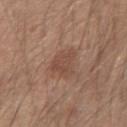Clinical impression: Part of a total-body skin-imaging series; this lesion was reviewed on a skin check and was not flagged for biopsy. Acquisition and patient details: A male subject, aged 28 to 32. A region of skin cropped from a whole-body photographic capture, roughly 15 mm wide. Located on the left forearm.